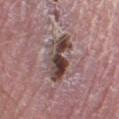Q: What kind of image is this?
A: ~15 mm tile from a whole-body skin photo
Q: Lesion size?
A: ≈6.5 mm
Q: What is the anatomic site?
A: the right lower leg
Q: Who is the patient?
A: male, about 40 years old